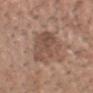Notes:
- workup — no biopsy performed (imaged during a skin exam)